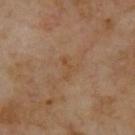notes = catalogued during a skin exam; not biopsied | site = the upper back | subject = male, aged 68 to 72 | lesion diameter = about 2.5 mm | image = total-body-photography crop, ~15 mm field of view.